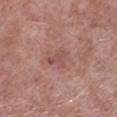notes=total-body-photography surveillance lesion; no biopsy